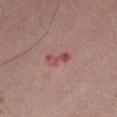Findings:
* biopsy status — total-body-photography surveillance lesion; no biopsy
* image source — ~15 mm crop, total-body skin-cancer survey
* subject — male, in their mid- to late 70s
* body site — the right thigh